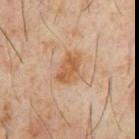notes = catalogued during a skin exam; not biopsied
body site = the abdomen
diameter = ≈4 mm
subject = male, aged 58 to 62
image = ~15 mm tile from a whole-body skin photo
automated lesion analysis = an area of roughly 8 mm², an eccentricity of roughly 0.75, and a symmetry-axis asymmetry near 0.3